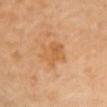Recorded during total-body skin imaging; not selected for excision or biopsy.
Cropped from a whole-body photographic skin survey; the tile spans about 15 mm.
The recorded lesion diameter is about 4 mm.
Captured under cross-polarized illumination.
A female patient, aged 53–57.
An algorithmic analysis of the crop reported an area of roughly 9.5 mm² and a shape-asymmetry score of about 0.3 (0 = symmetric). It also reported a normalized lesion–skin contrast near 5.5. The analysis additionally found a border-irregularity index near 3.5/10, internal color variation of about 3.5 on a 0–10 scale, and a peripheral color-asymmetry measure near 1. It also reported a nevus-likeness score of about 0/100.
The lesion is on the front of the torso.